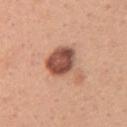<lesion>
<biopsy_status>not biopsied; imaged during a skin examination</biopsy_status>
<automated_metrics>
  <eccentricity>0.65</eccentricity>
  <cielab_L>53</cielab_L>
  <cielab_a>24</cielab_a>
  <cielab_b>29</cielab_b>
  <vs_skin_contrast_norm>11.0</vs_skin_contrast_norm>
  <nevus_likeness_0_100>55</nevus_likeness_0_100>
</automated_metrics>
<site>left upper arm</site>
<image>
  <source>total-body photography crop</source>
  <field_of_view_mm>15</field_of_view_mm>
</image>
<lesion_size>
  <long_diameter_mm_approx>5.0</long_diameter_mm_approx>
</lesion_size>
<patient>
  <sex>female</sex>
  <age_approx>30</age_approx>
</patient>
</lesion>